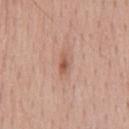workup: no biopsy performed (imaged during a skin exam) | body site: the chest | patient: male, approximately 55 years of age | image-analysis metrics: a lesion area of about 3 mm², an outline eccentricity of about 0.9 (0 = round, 1 = elongated), and a symmetry-axis asymmetry near 0.25; border irregularity of about 2.5 on a 0–10 scale and radial color variation of about 1 | image source: 15 mm crop, total-body photography.